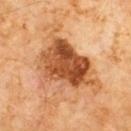{"biopsy_status": "not biopsied; imaged during a skin examination", "image": {"source": "total-body photography crop", "field_of_view_mm": 15}, "patient": {"sex": "male", "age_approx": 60}, "site": "chest"}